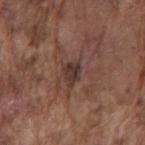Q: Is there a histopathology result?
A: imaged on a skin check; not biopsied
Q: What is the anatomic site?
A: the mid back
Q: Automated lesion metrics?
A: a lesion color around L≈35 a*≈18 b*≈20 in CIELAB, a lesion–skin lightness drop of about 9, and a normalized border contrast of about 9; a classifier nevus-likeness of about 5/100 and a lesion-detection confidence of about 100/100
Q: What is the imaging modality?
A: 15 mm crop, total-body photography
Q: Lesion size?
A: about 3 mm
Q: Patient demographics?
A: male, in their mid- to late 70s